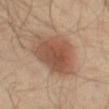<case>
<biopsy_status>not biopsied; imaged during a skin examination</biopsy_status>
</case>